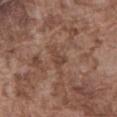The lesion was photographed on a routine skin check and not biopsied; there is no pathology result. A male subject, aged around 75. On the abdomen. The lesion-visualizer software estimated an area of roughly 3.5 mm², a shape eccentricity near 0.8, and a shape-asymmetry score of about 0.55 (0 = symmetric). It also reported a border-irregularity rating of about 5.5/10, a color-variation rating of about 1/10, and peripheral color asymmetry of about 0.5. The software also gave a classifier nevus-likeness of about 0/100 and a lesion-detection confidence of about 100/100. This image is a 15 mm lesion crop taken from a total-body photograph.